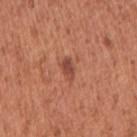biopsy_status: not biopsied; imaged during a skin examination
patient:
  sex: female
  age_approx: 50
image:
  source: total-body photography crop
  field_of_view_mm: 15
lighting: white-light
automated_metrics:
  area_mm2_approx: 3.5
  shape_asymmetry: 0.2
  border_irregularity_0_10: 2.0
  color_variation_0_10: 2.5
  peripheral_color_asymmetry: 1.0
  lesion_detection_confidence_0_100: 100
site: right upper arm
lesion_size:
  long_diameter_mm_approx: 2.5